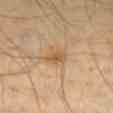No biopsy was performed on this lesion — it was imaged during a full skin examination and was not determined to be concerning.
The lesion's longest dimension is about 3 mm.
A male subject, about 40 years old.
Cropped from a whole-body photographic skin survey; the tile spans about 15 mm.
Located on the chest.
Captured under cross-polarized illumination.